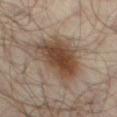Notes:
* follow-up · no biopsy performed (imaged during a skin exam)
* size · ≈7 mm
* site · the left thigh
* illumination · cross-polarized
* patient · male, about 65 years old
* image · ~15 mm crop, total-body skin-cancer survey